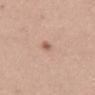Assessment: No biopsy was performed on this lesion — it was imaged during a full skin examination and was not determined to be concerning. Acquisition and patient details: Captured under white-light illumination. About 3 mm across. A region of skin cropped from a whole-body photographic capture, roughly 15 mm wide. From the mid back. A female subject, aged approximately 35.